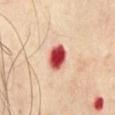- follow-up · imaged on a skin check; not biopsied
- tile lighting · cross-polarized illumination
- image source · 15 mm crop, total-body photography
- location · the chest
- image-analysis metrics · a lesion color around L≈51 a*≈39 b*≈29 in CIELAB, roughly 24 lightness units darker than nearby skin, and a normalized border contrast of about 15
- subject · male, about 70 years old